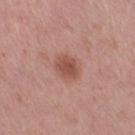Impression: The lesion was photographed on a routine skin check and not biopsied; there is no pathology result. Clinical summary: A female subject, aged 53 to 57. A close-up tile cropped from a whole-body skin photograph, about 15 mm across. The lesion is on the right thigh.